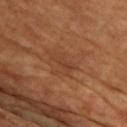workup=no biopsy performed (imaged during a skin exam); lesion diameter=about 3.5 mm; site=the front of the torso; acquisition=~15 mm tile from a whole-body skin photo; image-analysis metrics=a border-irregularity rating of about 4.5/10, internal color variation of about 0 on a 0–10 scale, and peripheral color asymmetry of about 0; patient=male, aged around 65; illumination=cross-polarized illumination.